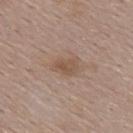A region of skin cropped from a whole-body photographic capture, roughly 15 mm wide.
Approximately 3 mm at its widest.
Automated tile analysis of the lesion measured a lesion–skin lightness drop of about 8 and a normalized lesion–skin contrast near 6. The analysis additionally found an automated nevus-likeness rating near 0 out of 100 and lesion-presence confidence of about 100/100.
A female subject, aged 58 to 62.
From the upper back.